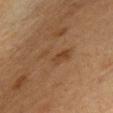notes: no biopsy performed (imaged during a skin exam); anatomic site: the chest; acquisition: ~15 mm tile from a whole-body skin photo; lighting: cross-polarized illumination; patient: male, aged 63–67; image-analysis metrics: a normalized lesion–skin contrast near 5.